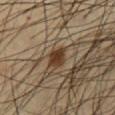The lesion was photographed on a routine skin check and not biopsied; there is no pathology result. From the abdomen. The tile uses cross-polarized illumination. Approximately 2.5 mm at its widest. The subject is a male in their 40s. An algorithmic analysis of the crop reported an area of roughly 5 mm² and two-axis asymmetry of about 0.3. The analysis additionally found an average lesion color of about L≈38 a*≈15 b*≈30 (CIELAB) and roughly 12 lightness units darker than nearby skin. And it measured a peripheral color-asymmetry measure near 0.5. It also reported an automated nevus-likeness rating near 100 out of 100 and a detector confidence of about 100 out of 100 that the crop contains a lesion. Cropped from a total-body skin-imaging series; the visible field is about 15 mm.